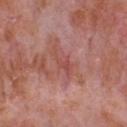No biopsy was performed on this lesion — it was imaged during a full skin examination and was not determined to be concerning.
The lesion-visualizer software estimated a mean CIELAB color near L≈50 a*≈27 b*≈25, a lesion–skin lightness drop of about 7, and a lesion-to-skin contrast of about 5 (normalized; higher = more distinct).
A 15 mm close-up extracted from a 3D total-body photography capture.
The lesion is located on the front of the torso.
A male patient, in their mid-60s.
The tile uses white-light illumination.